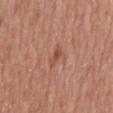biopsy_status: not biopsied; imaged during a skin examination
patient:
  sex: male
  age_approx: 65
image:
  source: total-body photography crop
  field_of_view_mm: 15
site: chest